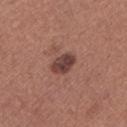Image and clinical context:
Located on the left lower leg. An algorithmic analysis of the crop reported an outline eccentricity of about 0.75 (0 = round, 1 = elongated). The analysis additionally found an average lesion color of about L≈41 a*≈21 b*≈22 (CIELAB) and about 12 CIELAB-L* units darker than the surrounding skin. It also reported lesion-presence confidence of about 100/100. A female subject in their 50s. The recorded lesion diameter is about 3.5 mm. A 15 mm close-up extracted from a 3D total-body photography capture.